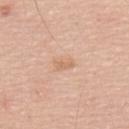Assessment:
No biopsy was performed on this lesion — it was imaged during a full skin examination and was not determined to be concerning.
Image and clinical context:
An algorithmic analysis of the crop reported a footprint of about 2.5 mm², an outline eccentricity of about 0.9 (0 = round, 1 = elongated), and a symmetry-axis asymmetry near 0.3. The software also gave an average lesion color of about L≈66 a*≈20 b*≈34 (CIELAB) and roughly 7 lightness units darker than nearby skin. And it measured a classifier nevus-likeness of about 0/100 and a lesion-detection confidence of about 100/100. A male patient aged 68–72. A close-up tile cropped from a whole-body skin photograph, about 15 mm across. The tile uses white-light illumination. Located on the upper back.